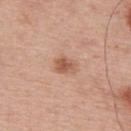Notes:
• biopsy status · total-body-photography surveillance lesion; no biopsy
• illumination · white-light illumination
• automated metrics · a lesion color around L≈57 a*≈22 b*≈32 in CIELAB, about 10 CIELAB-L* units darker than the surrounding skin, and a normalized lesion–skin contrast near 7.5; border irregularity of about 2.5 on a 0–10 scale and peripheral color asymmetry of about 1; a nevus-likeness score of about 70/100 and a detector confidence of about 100 out of 100 that the crop contains a lesion
• subject · male, aged around 65
• image source · ~15 mm tile from a whole-body skin photo
• anatomic site · the upper back
• lesion diameter · about 2.5 mm